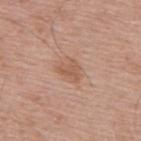Part of a total-body skin-imaging series; this lesion was reviewed on a skin check and was not flagged for biopsy. Captured under white-light illumination. This image is a 15 mm lesion crop taken from a total-body photograph. The lesion is on the mid back. The subject is a male aged approximately 55. Automated tile analysis of the lesion measured border irregularity of about 4 on a 0–10 scale and a color-variation rating of about 1.5/10. Approximately 3 mm at its widest.